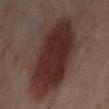Context: The lesion is on the left lower leg. A female subject aged 53 to 57. Cropped from a total-body skin-imaging series; the visible field is about 15 mm. This is a cross-polarized tile. About 14 mm across. An algorithmic analysis of the crop reported border irregularity of about 3.5 on a 0–10 scale, a within-lesion color-variation index near 5.5/10, and peripheral color asymmetry of about 1.5. The analysis additionally found a nevus-likeness score of about 100/100 and a lesion-detection confidence of about 100/100.